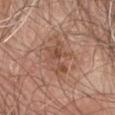Clinical impression:
Part of a total-body skin-imaging series; this lesion was reviewed on a skin check and was not flagged for biopsy.
Image and clinical context:
The subject is a male about 80 years old. The lesion is on the abdomen. This is a white-light tile. Cropped from a total-body skin-imaging series; the visible field is about 15 mm.